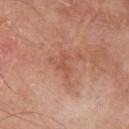notes: catalogued during a skin exam; not biopsied
automated metrics: an area of roughly 2.5 mm², an eccentricity of roughly 0.85, and a shape-asymmetry score of about 0.65 (0 = symmetric)
image source: ~15 mm tile from a whole-body skin photo
subject: male, aged 78 to 82
body site: the arm